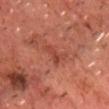follow-up: no biopsy performed (imaged during a skin exam) | location: the chest | illumination: cross-polarized illumination | lesion size: ≈2.5 mm | TBP lesion metrics: an area of roughly 3.5 mm² and an eccentricity of roughly 0.7; an average lesion color of about L≈43 a*≈30 b*≈30 (CIELAB), about 7 CIELAB-L* units darker than the surrounding skin, and a lesion-to-skin contrast of about 5.5 (normalized; higher = more distinct) | image: 15 mm crop, total-body photography | patient: male, aged 68–72.